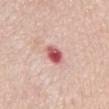Assessment: No biopsy was performed on this lesion — it was imaged during a full skin examination and was not determined to be concerning. Acquisition and patient details: From the chest. A 15 mm crop from a total-body photograph taken for skin-cancer surveillance. A female subject, in their mid-60s. The lesion's longest dimension is about 2.5 mm.